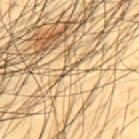Recorded during total-body skin imaging; not selected for excision or biopsy.
A close-up tile cropped from a whole-body skin photograph, about 15 mm across.
The lesion is located on the back.
The subject is a male aged 43–47.